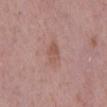Q: Was a biopsy performed?
A: no biopsy performed (imaged during a skin exam)
Q: How was the tile lit?
A: white-light illumination
Q: Where on the body is the lesion?
A: the mid back
Q: What did automated image analysis measure?
A: an average lesion color of about L≈54 a*≈22 b*≈25 (CIELAB), about 7 CIELAB-L* units darker than the surrounding skin, and a normalized lesion–skin contrast near 5.5; border irregularity of about 3 on a 0–10 scale, a color-variation rating of about 3/10, and peripheral color asymmetry of about 1
Q: Who is the patient?
A: male, in their 60s
Q: Lesion size?
A: about 3.5 mm
Q: How was this image acquired?
A: 15 mm crop, total-body photography